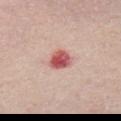Assessment: Imaged during a routine full-body skin examination; the lesion was not biopsied and no histopathology is available. Image and clinical context: The lesion-visualizer software estimated a footprint of about 5.5 mm², an outline eccentricity of about 0.55 (0 = round, 1 = elongated), and a shape-asymmetry score of about 0.25 (0 = symmetric). The tile uses white-light illumination. The lesion's longest dimension is about 2.5 mm. The lesion is located on the chest. A female patient, aged 68–72. A region of skin cropped from a whole-body photographic capture, roughly 15 mm wide.